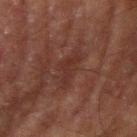Findings:
• lighting — cross-polarized illumination
• size — about 4 mm
• subject — male, approximately 70 years of age
• anatomic site — the right upper arm
• image source — total-body-photography crop, ~15 mm field of view